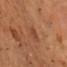Case summary:
- workup — catalogued during a skin exam; not biopsied
- diameter — about 3.5 mm
- patient — male, in their 60s
- site — the chest
- image-analysis metrics — an area of roughly 4.5 mm², an outline eccentricity of about 0.85 (0 = round, 1 = elongated), and two-axis asymmetry of about 0.3; a border-irregularity rating of about 3.5/10, a color-variation rating of about 2.5/10, and a peripheral color-asymmetry measure near 1
- imaging modality — ~15 mm crop, total-body skin-cancer survey
- tile lighting — cross-polarized